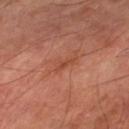Q: Is there a histopathology result?
A: total-body-photography surveillance lesion; no biopsy
Q: What is the anatomic site?
A: the left thigh
Q: What is the imaging modality?
A: ~15 mm tile from a whole-body skin photo
Q: What did automated image analysis measure?
A: an area of roughly 2 mm² and an outline eccentricity of about 0.95 (0 = round, 1 = elongated)
Q: Who is the patient?
A: male, approximately 70 years of age
Q: What is the lesion's diameter?
A: ≈2.5 mm